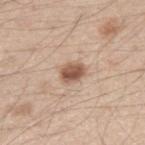This lesion was catalogued during total-body skin photography and was not selected for biopsy.